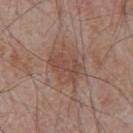follow-up — no biopsy performed (imaged during a skin exam) | image source — 15 mm crop, total-body photography | subject — male, aged 68–72 | anatomic site — the chest.